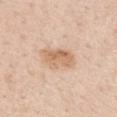  biopsy_status: not biopsied; imaged during a skin examination
  patient:
    sex: female
    age_approx: 45
  lighting: white-light
  image:
    source: total-body photography crop
    field_of_view_mm: 15
  site: mid back
  automated_metrics:
    nevus_likeness_0_100: 25